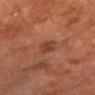Captured during whole-body skin photography for melanoma surveillance; the lesion was not biopsied. The recorded lesion diameter is about 2.5 mm. The total-body-photography lesion software estimated an area of roughly 4 mm² and an outline eccentricity of about 0.75 (0 = round, 1 = elongated). And it measured a lesion–skin lightness drop of about 7. And it measured an automated nevus-likeness rating near 5 out of 100 and lesion-presence confidence of about 100/100. The patient is a male aged around 75. A lesion tile, about 15 mm wide, cut from a 3D total-body photograph. The lesion is located on the chest. Captured under cross-polarized illumination.